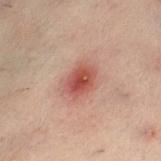Assessment: The lesion was tiled from a total-body skin photograph and was not biopsied. Image and clinical context: Cropped from a whole-body photographic skin survey; the tile spans about 15 mm. The patient is a female in their mid- to late 50s. The lesion is on the left lower leg. Imaged with cross-polarized lighting. Approximately 3.5 mm at its widest.